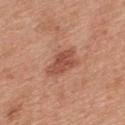- biopsy status · catalogued during a skin exam; not biopsied
- tile lighting · white-light illumination
- image · total-body-photography crop, ~15 mm field of view
- site · the upper back
- image-analysis metrics · a footprint of about 8 mm²; an average lesion color of about L≈51 a*≈26 b*≈31 (CIELAB) and a normalized lesion–skin contrast near 7.5; a border-irregularity index near 3/10
- subject · male, in their 30s